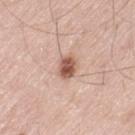Clinical impression: Captured during whole-body skin photography for melanoma surveillance; the lesion was not biopsied. Image and clinical context: The lesion-visualizer software estimated about 16 CIELAB-L* units darker than the surrounding skin and a lesion-to-skin contrast of about 10 (normalized; higher = more distinct). Located on the left thigh. A region of skin cropped from a whole-body photographic capture, roughly 15 mm wide. The lesion's longest dimension is about 3 mm. A male subject approximately 80 years of age.